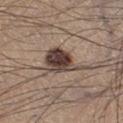Clinical impression:
The lesion was tiled from a total-body skin photograph and was not biopsied.
Context:
Measured at roughly 5.5 mm in maximum diameter. This is a white-light tile. The lesion is on the left thigh. A male subject in their mid- to late 30s. A 15 mm crop from a total-body photograph taken for skin-cancer surveillance.